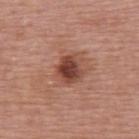follow-up: imaged on a skin check; not biopsied
subject: female, aged 63 to 67
body site: the upper back
image source: ~15 mm crop, total-body skin-cancer survey